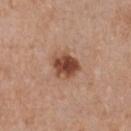Part of a total-body skin-imaging series; this lesion was reviewed on a skin check and was not flagged for biopsy.
A female patient, approximately 35 years of age.
The lesion is on the chest.
This is a white-light tile.
Automated image analysis of the tile measured an outline eccentricity of about 0.55 (0 = round, 1 = elongated). And it measured an average lesion color of about L≈46 a*≈23 b*≈30 (CIELAB), roughly 15 lightness units darker than nearby skin, and a normalized border contrast of about 11. The software also gave a border-irregularity index near 2/10 and peripheral color asymmetry of about 1.5. The software also gave a detector confidence of about 100 out of 100 that the crop contains a lesion.
A region of skin cropped from a whole-body photographic capture, roughly 15 mm wide.
Measured at roughly 3.5 mm in maximum diameter.